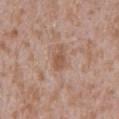On the mid back. Automated image analysis of the tile measured a mean CIELAB color near L≈54 a*≈19 b*≈30 and roughly 9 lightness units darker than nearby skin. The analysis additionally found a classifier nevus-likeness of about 0/100 and a detector confidence of about 100 out of 100 that the crop contains a lesion. A male patient, approximately 45 years of age. A 15 mm crop from a total-body photograph taken for skin-cancer surveillance. Measured at roughly 3.5 mm in maximum diameter. Captured under white-light illumination.